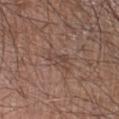{
  "biopsy_status": "not biopsied; imaged during a skin examination",
  "automated_metrics": {
    "vs_skin_darker_L": 7.0,
    "vs_skin_contrast_norm": 5.5
  },
  "site": "leg",
  "lighting": "white-light",
  "patient": {
    "sex": "male",
    "age_approx": 60
  },
  "image": {
    "source": "total-body photography crop",
    "field_of_view_mm": 15
  },
  "lesion_size": {
    "long_diameter_mm_approx": 2.5
  }
}